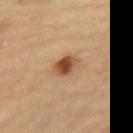Part of a total-body skin-imaging series; this lesion was reviewed on a skin check and was not flagged for biopsy.